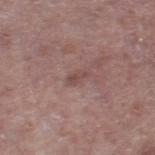notes=no biopsy performed (imaged during a skin exam)
subject=male, in their 70s
illumination=white-light illumination
automated metrics=a lesion color around L≈47 a*≈20 b*≈21 in CIELAB, roughly 7 lightness units darker than nearby skin, and a normalized lesion–skin contrast near 5.5; border irregularity of about 4 on a 0–10 scale, internal color variation of about 0 on a 0–10 scale, and radial color variation of about 0; an automated nevus-likeness rating near 0 out of 100 and a detector confidence of about 100 out of 100 that the crop contains a lesion
site=the right thigh
image=15 mm crop, total-body photography